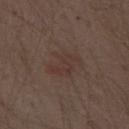follow-up: no biopsy performed (imaged during a skin exam)
lesion size: ≈4 mm
subject: male, aged 68 to 72
TBP lesion metrics: a footprint of about 8.5 mm², a shape eccentricity near 0.65, and two-axis asymmetry of about 0.3; an automated nevus-likeness rating near 10 out of 100 and lesion-presence confidence of about 100/100
location: the abdomen
acquisition: ~15 mm crop, total-body skin-cancer survey
tile lighting: white-light illumination